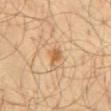Captured during whole-body skin photography for melanoma surveillance; the lesion was not biopsied. The patient is a male aged 53–57. A roughly 15 mm field-of-view crop from a total-body skin photograph. The lesion's longest dimension is about 2 mm. The lesion-visualizer software estimated an average lesion color of about L≈60 a*≈21 b*≈40 (CIELAB), a lesion–skin lightness drop of about 10, and a normalized lesion–skin contrast near 7.5. The analysis additionally found radial color variation of about 0.5. It also reported a nevus-likeness score of about 85/100. Located on the lower back. Captured under cross-polarized illumination.